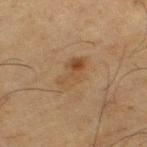Q: Was this lesion biopsied?
A: no biopsy performed (imaged during a skin exam)
Q: What is the imaging modality?
A: 15 mm crop, total-body photography
Q: What is the anatomic site?
A: the leg
Q: Who is the patient?
A: male, aged 63–67